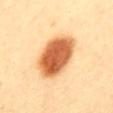  patient:
    sex: female
    age_approx: 40
  site: mid back
  image:
    source: total-body photography crop
    field_of_view_mm: 15
  lighting: cross-polarized